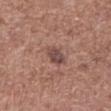Impression:
Part of a total-body skin-imaging series; this lesion was reviewed on a skin check and was not flagged for biopsy.
Clinical summary:
The tile uses white-light illumination. From the front of the torso. A male patient, in their mid-70s. A 15 mm close-up tile from a total-body photography series done for melanoma screening. The recorded lesion diameter is about 3 mm. Automated image analysis of the tile measured a footprint of about 5 mm² and two-axis asymmetry of about 0.15. And it measured a lesion–skin lightness drop of about 10 and a normalized lesion–skin contrast near 8. And it measured border irregularity of about 2 on a 0–10 scale. And it measured a classifier nevus-likeness of about 15/100.